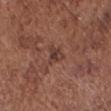• follow-up — catalogued during a skin exam; not biopsied
• lesion diameter — ≈3 mm
• body site — the head or neck
• subject — male, about 75 years old
• image — ~15 mm tile from a whole-body skin photo
• image-analysis metrics — a footprint of about 4 mm², an outline eccentricity of about 0.65 (0 = round, 1 = elongated), and a symmetry-axis asymmetry near 0.45; a border-irregularity rating of about 5/10, internal color variation of about 2 on a 0–10 scale, and radial color variation of about 0.5
• lighting — white-light illumination